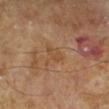Part of a total-body skin-imaging series; this lesion was reviewed on a skin check and was not flagged for biopsy.
The tile uses cross-polarized illumination.
The subject is a male aged around 65.
The lesion's longest dimension is about 3 mm.
Automated image analysis of the tile measured a mean CIELAB color near L≈46 a*≈18 b*≈33, about 5 CIELAB-L* units darker than the surrounding skin, and a normalized border contrast of about 5.
A 15 mm close-up extracted from a 3D total-body photography capture.